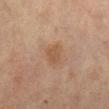Captured during whole-body skin photography for melanoma surveillance; the lesion was not biopsied. This image is a 15 mm lesion crop taken from a total-body photograph. Measured at roughly 2.5 mm in maximum diameter. The subject is a female in their 70s. The tile uses cross-polarized illumination. Located on the leg.